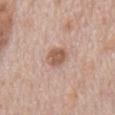notes: catalogued during a skin exam; not biopsied | patient: male, about 70 years old | lighting: white-light | TBP lesion metrics: a footprint of about 5.5 mm², an eccentricity of roughly 0.5, and a symmetry-axis asymmetry near 0.15; a border-irregularity rating of about 1.5/10, a color-variation rating of about 2/10, and peripheral color asymmetry of about 0.5 | imaging modality: total-body-photography crop, ~15 mm field of view | size: about 3 mm | body site: the abdomen.